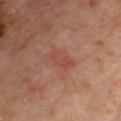– biopsy status — total-body-photography surveillance lesion; no biopsy
– anatomic site — the chest
– imaging modality — total-body-photography crop, ~15 mm field of view
– patient — male, in their mid-50s